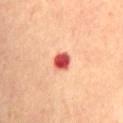<case>
  <biopsy_status>not biopsied; imaged during a skin examination</biopsy_status>
  <site>front of the torso</site>
  <image>
    <source>total-body photography crop</source>
    <field_of_view_mm>15</field_of_view_mm>
  </image>
  <automated_metrics>
    <area_mm2_approx>4.5</area_mm2_approx>
    <shape_asymmetry>0.2</shape_asymmetry>
    <cielab_L>55</cielab_L>
    <cielab_a>40</cielab_a>
    <cielab_b>33</cielab_b>
    <vs_skin_darker_L>20.0</vs_skin_darker_L>
    <vs_skin_contrast_norm>12.5</vs_skin_contrast_norm>
    <color_variation_0_10>6.0</color_variation_0_10>
  </automated_metrics>
  <lesion_size>
    <long_diameter_mm_approx>2.5</long_diameter_mm_approx>
  </lesion_size>
  <patient>
    <sex>female</sex>
    <age_approx>70</age_approx>
  </patient>
</case>